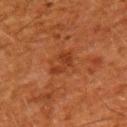Assessment:
The lesion was tiled from a total-body skin photograph and was not biopsied.
Context:
On the upper back. A male patient aged 58 to 62. About 3.5 mm across. A lesion tile, about 15 mm wide, cut from a 3D total-body photograph. Captured under cross-polarized illumination. The total-body-photography lesion software estimated an area of roughly 4.5 mm² and a symmetry-axis asymmetry near 0.45.